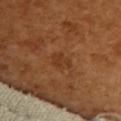workup = no biopsy performed (imaged during a skin exam); anatomic site = the upper back; imaging modality = 15 mm crop, total-body photography; patient = female, aged approximately 55.